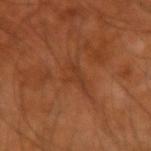{"biopsy_status": "not biopsied; imaged during a skin examination", "patient": {"sex": "male", "age_approx": 50}, "automated_metrics": {"eccentricity": 0.85, "shape_asymmetry": 0.65, "cielab_L": 37, "cielab_a": 24, "cielab_b": 34, "vs_skin_contrast_norm": 4.5, "border_irregularity_0_10": 7.0, "color_variation_0_10": 0.0, "nevus_likeness_0_100": 0, "lesion_detection_confidence_0_100": 60}, "site": "left arm", "image": {"source": "total-body photography crop", "field_of_view_mm": 15}, "lesion_size": {"long_diameter_mm_approx": 3.5}, "lighting": "cross-polarized"}